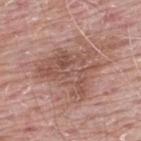Assessment: This lesion was catalogued during total-body skin photography and was not selected for biopsy. Background: Imaged with white-light lighting. A male patient, about 65 years old. The lesion is located on the upper back. A lesion tile, about 15 mm wide, cut from a 3D total-body photograph.